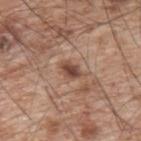Part of a total-body skin-imaging series; this lesion was reviewed on a skin check and was not flagged for biopsy. Approximately 2.5 mm at its widest. The subject is a male aged 63–67. Cropped from a total-body skin-imaging series; the visible field is about 15 mm. On the upper back.